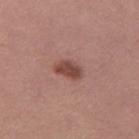acquisition: 15 mm crop, total-body photography; body site: the left thigh; subject: female, aged approximately 35; diameter: about 3.5 mm; tile lighting: white-light illumination.